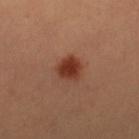notes: no biopsy performed (imaged during a skin exam)
anatomic site: the right lower leg
lesion diameter: ~3 mm (longest diameter)
patient: female, about 35 years old
lighting: cross-polarized
image source: ~15 mm tile from a whole-body skin photo